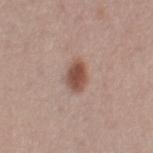Q: Was a biopsy performed?
A: imaged on a skin check; not biopsied
Q: What is the imaging modality?
A: ~15 mm crop, total-body skin-cancer survey
Q: What lighting was used for the tile?
A: white-light illumination
Q: What is the anatomic site?
A: the right thigh
Q: Patient demographics?
A: female, aged approximately 30